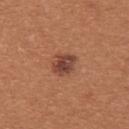Clinical impression: The lesion was tiled from a total-body skin photograph and was not biopsied. Image and clinical context: A lesion tile, about 15 mm wide, cut from a 3D total-body photograph. Captured under white-light illumination. A female patient about 40 years old. The lesion is located on the back.